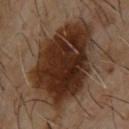biopsy_status: not biopsied; imaged during a skin examination
site: upper back
lighting: cross-polarized
automated_metrics:
  area_mm2_approx: 55.0
  eccentricity: 0.75
  shape_asymmetry: 0.15
  nevus_likeness_0_100: 80
  lesion_detection_confidence_0_100: 100
lesion_size:
  long_diameter_mm_approx: 11.0
patient:
  sex: male
  age_approx: 65
image:
  source: total-body photography crop
  field_of_view_mm: 15